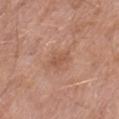Captured during whole-body skin photography for melanoma surveillance; the lesion was not biopsied. A male patient, in their mid- to late 60s. From the right thigh. Cropped from a total-body skin-imaging series; the visible field is about 15 mm.